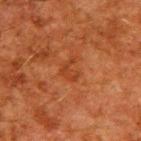Part of a total-body skin-imaging series; this lesion was reviewed on a skin check and was not flagged for biopsy. A close-up tile cropped from a whole-body skin photograph, about 15 mm across. Imaged with cross-polarized lighting. A male patient, in their 60s. The lesion is located on the upper back.